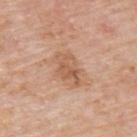The lesion was tiled from a total-body skin photograph and was not biopsied. A male subject aged 73–77. Captured under white-light illumination. From the upper back. The total-body-photography lesion software estimated a footprint of about 7.5 mm² and an eccentricity of roughly 0.8. The analysis additionally found border irregularity of about 4 on a 0–10 scale, internal color variation of about 4.5 on a 0–10 scale, and a peripheral color-asymmetry measure near 1.5. A 15 mm crop from a total-body photograph taken for skin-cancer surveillance. About 4 mm across.